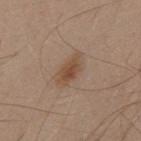Imaged during a routine full-body skin examination; the lesion was not biopsied and no histopathology is available.
The lesion-visualizer software estimated border irregularity of about 3 on a 0–10 scale, internal color variation of about 3.5 on a 0–10 scale, and a peripheral color-asymmetry measure near 1.
This is a white-light tile.
The patient is a male aged 28 to 32.
Cropped from a whole-body photographic skin survey; the tile spans about 15 mm.
The lesion is located on the upper back.